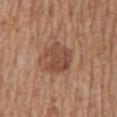notes: imaged on a skin check; not biopsied | lighting: white-light illumination | location: the mid back | automated metrics: an average lesion color of about L≈47 a*≈21 b*≈29 (CIELAB); a border-irregularity index near 3.5/10, internal color variation of about 3 on a 0–10 scale, and a peripheral color-asymmetry measure near 1; lesion-presence confidence of about 100/100 | size: ≈3.5 mm | patient: male, aged 68–72 | acquisition: total-body-photography crop, ~15 mm field of view.